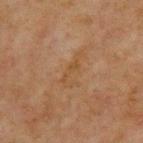Part of a total-body skin-imaging series; this lesion was reviewed on a skin check and was not flagged for biopsy. The subject is a male in their mid-60s. The tile uses cross-polarized illumination. A 15 mm close-up tile from a total-body photography series done for melanoma screening. The lesion-visualizer software estimated a footprint of about 4.5 mm² and a symmetry-axis asymmetry near 0.5. The software also gave a lesion color around L≈39 a*≈15 b*≈30 in CIELAB, roughly 4 lightness units darker than nearby skin, and a normalized border contrast of about 5. And it measured a within-lesion color-variation index near 0/10 and radial color variation of about 0. And it measured a classifier nevus-likeness of about 0/100 and lesion-presence confidence of about 100/100. On the chest. Approximately 4 mm at its widest.